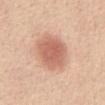Clinical impression:
The lesion was tiled from a total-body skin photograph and was not biopsied.
Acquisition and patient details:
This is a white-light tile. The recorded lesion diameter is about 4.5 mm. A female patient, aged 48 to 52. A close-up tile cropped from a whole-body skin photograph, about 15 mm across. From the abdomen.